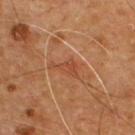Notes:
– biopsy status — total-body-photography surveillance lesion; no biopsy
– image — ~15 mm crop, total-body skin-cancer survey
– patient — male, roughly 55 years of age
– location — the chest